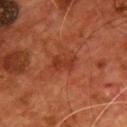follow-up: catalogued during a skin exam; not biopsied
body site: the chest
acquisition: ~15 mm tile from a whole-body skin photo
patient: male, aged approximately 55
lesion size: ~2.5 mm (longest diameter)
lighting: cross-polarized illumination